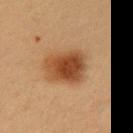Captured during whole-body skin photography for melanoma surveillance; the lesion was not biopsied. An algorithmic analysis of the crop reported a lesion area of about 14 mm², an outline eccentricity of about 0.5 (0 = round, 1 = elongated), and a symmetry-axis asymmetry near 0.2. On the left upper arm. The subject is a female approximately 40 years of age. A 15 mm close-up tile from a total-body photography series done for melanoma screening. Approximately 4.5 mm at its widest.